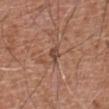Part of a total-body skin-imaging series; this lesion was reviewed on a skin check and was not flagged for biopsy.
The patient is a male roughly 75 years of age.
An algorithmic analysis of the crop reported a border-irregularity rating of about 4/10 and a color-variation rating of about 2/10. It also reported a classifier nevus-likeness of about 0/100 and a lesion-detection confidence of about 85/100.
Imaged with white-light lighting.
A 15 mm close-up tile from a total-body photography series done for melanoma screening.
The recorded lesion diameter is about 2.5 mm.
The lesion is on the front of the torso.